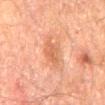This lesion was catalogued during total-body skin photography and was not selected for biopsy. The tile uses cross-polarized illumination. A 15 mm close-up tile from a total-body photography series done for melanoma screening. A male subject, about 60 years old. The lesion is located on the back. Longest diameter approximately 3.5 mm.